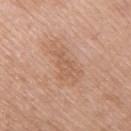Q: Was a biopsy performed?
A: imaged on a skin check; not biopsied
Q: How was the tile lit?
A: white-light illumination
Q: What is the imaging modality?
A: ~15 mm crop, total-body skin-cancer survey
Q: Lesion location?
A: the back
Q: What is the lesion's diameter?
A: ~4 mm (longest diameter)
Q: Who is the patient?
A: male, aged approximately 70